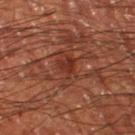Assessment:
This lesion was catalogued during total-body skin photography and was not selected for biopsy.
Context:
The lesion is on the left thigh. A male subject about 60 years old. The total-body-photography lesion software estimated an area of roughly 9 mm², an eccentricity of roughly 0.8, and two-axis asymmetry of about 0.25. And it measured roughly 7 lightness units darker than nearby skin and a normalized border contrast of about 6.5. And it measured an automated nevus-likeness rating near 0 out of 100 and a detector confidence of about 70 out of 100 that the crop contains a lesion. This image is a 15 mm lesion crop taken from a total-body photograph. Imaged with cross-polarized lighting.